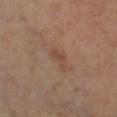follow-up: no biopsy performed (imaged during a skin exam)
lighting: cross-polarized illumination
patient: male, in their mid- to late 50s
size: ≈3 mm
site: the right lower leg
image-analysis metrics: a lesion area of about 3.5 mm², a shape eccentricity near 0.9, and a symmetry-axis asymmetry near 0.55; a mean CIELAB color near L≈44 a*≈19 b*≈28, roughly 6 lightness units darker than nearby skin, and a normalized border contrast of about 5.5; a border-irregularity index near 5.5/10, a within-lesion color-variation index near 0.5/10, and peripheral color asymmetry of about 0
acquisition: 15 mm crop, total-body photography